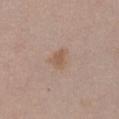| feature | finding |
|---|---|
| follow-up | catalogued during a skin exam; not biopsied |
| patient | female, roughly 30 years of age |
| location | the front of the torso |
| lighting | white-light illumination |
| image | ~15 mm crop, total-body skin-cancer survey |
| automated lesion analysis | an area of roughly 4.5 mm² and a symmetry-axis asymmetry near 0.35; an automated nevus-likeness rating near 20 out of 100 and lesion-presence confidence of about 100/100 |